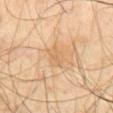Findings:
- follow-up · imaged on a skin check; not biopsied
- imaging modality · ~15 mm tile from a whole-body skin photo
- image-analysis metrics · a lesion area of about 5 mm², a shape eccentricity near 0.85, and a shape-asymmetry score of about 0.25 (0 = symmetric); border irregularity of about 3 on a 0–10 scale, a color-variation rating of about 2/10, and radial color variation of about 0.5
- diameter · ≈3.5 mm
- body site · the abdomen
- subject · male, aged around 55
- tile lighting · cross-polarized illumination